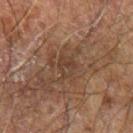Recorded during total-body skin imaging; not selected for excision or biopsy. The recorded lesion diameter is about 2.5 mm. An algorithmic analysis of the crop reported a footprint of about 4.5 mm², an eccentricity of roughly 0.6, and two-axis asymmetry of about 0.2. It also reported a mean CIELAB color near L≈31 a*≈14 b*≈23 and roughly 5 lightness units darker than nearby skin. It also reported an automated nevus-likeness rating near 0 out of 100 and lesion-presence confidence of about 65/100. Captured under cross-polarized illumination. A lesion tile, about 15 mm wide, cut from a 3D total-body photograph. On the arm. A male subject, roughly 70 years of age.